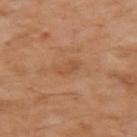Captured during whole-body skin photography for melanoma surveillance; the lesion was not biopsied. From the left upper arm. A lesion tile, about 15 mm wide, cut from a 3D total-body photograph. The patient is a male approximately 55 years of age.